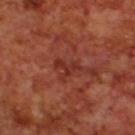| key | value |
|---|---|
| workup | no biopsy performed (imaged during a skin exam) |
| lesion size | ~3.5 mm (longest diameter) |
| imaging modality | total-body-photography crop, ~15 mm field of view |
| location | the upper back |
| subject | male, approximately 70 years of age |
| tile lighting | cross-polarized |
| automated lesion analysis | a lesion area of about 4 mm², an eccentricity of roughly 0.85, and a symmetry-axis asymmetry near 0.65; a lesion color around L≈31 a*≈29 b*≈29 in CIELAB, a lesion–skin lightness drop of about 7, and a normalized border contrast of about 6 |